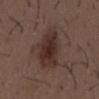Notes:
• follow-up · imaged on a skin check; not biopsied
• size · about 6 mm
• subject · male, in their 50s
• site · the mid back
• automated metrics · a mean CIELAB color near L≈30 a*≈17 b*≈21, a lesion–skin lightness drop of about 10, and a normalized lesion–skin contrast near 9.5; internal color variation of about 3.5 on a 0–10 scale and radial color variation of about 1; a detector confidence of about 100 out of 100 that the crop contains a lesion
• image · ~15 mm tile from a whole-body skin photo